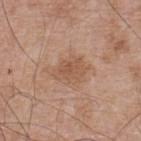site: the back | subject: male, in their mid- to late 60s | acquisition: ~15 mm tile from a whole-body skin photo | lighting: white-light.